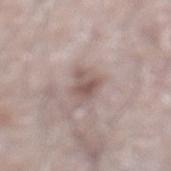Case summary:
- workup · catalogued during a skin exam; not biopsied